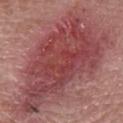Clinical impression:
The lesion was photographed on a routine skin check and not biopsied; there is no pathology result.
Acquisition and patient details:
The lesion's longest dimension is about 15 mm. Imaged with white-light lighting. The patient is a female aged approximately 60. A region of skin cropped from a whole-body photographic capture, roughly 15 mm wide. The lesion is located on the right forearm.